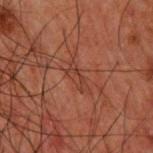Impression:
Recorded during total-body skin imaging; not selected for excision or biopsy.
Background:
The recorded lesion diameter is about 3 mm. On the upper back. A region of skin cropped from a whole-body photographic capture, roughly 15 mm wide. The tile uses cross-polarized illumination. The patient is a male roughly 50 years of age. The lesion-visualizer software estimated a detector confidence of about 80 out of 100 that the crop contains a lesion.